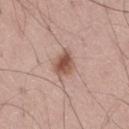Assessment: The lesion was photographed on a routine skin check and not biopsied; there is no pathology result. Background: This image is a 15 mm lesion crop taken from a total-body photograph. Automated image analysis of the tile measured a normalized lesion–skin contrast near 9. The software also gave an automated nevus-likeness rating near 95 out of 100 and a detector confidence of about 100 out of 100 that the crop contains a lesion. Captured under white-light illumination. A male subject, about 55 years old.